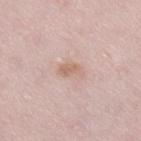The lesion was photographed on a routine skin check and not biopsied; there is no pathology result.
On the right thigh.
A female patient, roughly 30 years of age.
A lesion tile, about 15 mm wide, cut from a 3D total-body photograph.
The lesion-visualizer software estimated a footprint of about 3.5 mm² and a symmetry-axis asymmetry near 0.35. The software also gave a mean CIELAB color near L≈64 a*≈18 b*≈27, about 8 CIELAB-L* units darker than the surrounding skin, and a normalized border contrast of about 6. The analysis additionally found a border-irregularity rating of about 3.5/10, internal color variation of about 1 on a 0–10 scale, and peripheral color asymmetry of about 0.5.
The tile uses white-light illumination.